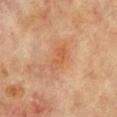Assessment:
No biopsy was performed on this lesion — it was imaged during a full skin examination and was not determined to be concerning.
Context:
On the chest. A close-up tile cropped from a whole-body skin photograph, about 15 mm across. Approximately 3.5 mm at its widest. A female patient, aged around 80.